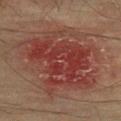Background:
An algorithmic analysis of the crop reported an average lesion color of about L≈33 a*≈22 b*≈22 (CIELAB), about 8 CIELAB-L* units darker than the surrounding skin, and a normalized lesion–skin contrast near 7.5. The software also gave a classifier nevus-likeness of about 0/100 and a lesion-detection confidence of about 100/100. A male patient, aged 73 to 77. The lesion is on the upper back. This is a cross-polarized tile. About 11.5 mm across. A roughly 15 mm field-of-view crop from a total-body skin photograph.